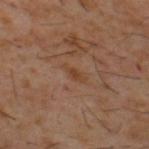imaging modality: ~15 mm crop, total-body skin-cancer survey | subject: male, aged approximately 60 | automated metrics: an area of roughly 2.5 mm², an outline eccentricity of about 0.9 (0 = round, 1 = elongated), and two-axis asymmetry of about 0.3; a classifier nevus-likeness of about 0/100 | lesion size: ≈3 mm | body site: the upper back.